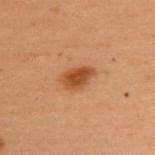<case>
<biopsy_status>not biopsied; imaged during a skin examination</biopsy_status>
<site>upper back</site>
<image>
  <source>total-body photography crop</source>
  <field_of_view_mm>15</field_of_view_mm>
</image>
<lighting>cross-polarized</lighting>
<automated_metrics>
  <area_mm2_approx>6.5</area_mm2_approx>
  <eccentricity>0.85</eccentricity>
  <nevus_likeness_0_100>100</nevus_likeness_0_100>
  <lesion_detection_confidence_0_100>100</lesion_detection_confidence_0_100>
</automated_metrics>
<patient>
  <sex>female</sex>
  <age_approx>50</age_approx>
</patient>
</case>